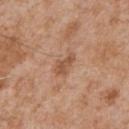Q: Is there a histopathology result?
A: total-body-photography surveillance lesion; no biopsy
Q: Lesion size?
A: ~3 mm (longest diameter)
Q: Patient demographics?
A: male, in their mid-60s
Q: How was this image acquired?
A: 15 mm crop, total-body photography
Q: Lesion location?
A: the chest